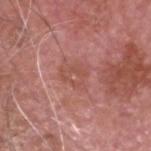subject=male, in their mid-70s | site=the head or neck | image source=15 mm crop, total-body photography.